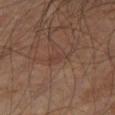Clinical impression: No biopsy was performed on this lesion — it was imaged during a full skin examination and was not determined to be concerning. Acquisition and patient details: Approximately 3 mm at its widest. Imaged with cross-polarized lighting. Located on the leg. The patient is a male in their 60s. Cropped from a whole-body photographic skin survey; the tile spans about 15 mm.